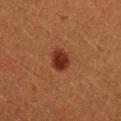Part of a total-body skin-imaging series; this lesion was reviewed on a skin check and was not flagged for biopsy.
From the right forearm.
The subject is a male approximately 40 years of age.
Cropped from a total-body skin-imaging series; the visible field is about 15 mm.
This is a cross-polarized tile.
The total-body-photography lesion software estimated a lesion area of about 6.5 mm², an outline eccentricity of about 0.4 (0 = round, 1 = elongated), and a symmetry-axis asymmetry near 0.15. The software also gave a nevus-likeness score of about 100/100.
The recorded lesion diameter is about 3 mm.